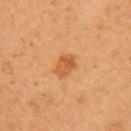Clinical impression:
No biopsy was performed on this lesion — it was imaged during a full skin examination and was not determined to be concerning.
Background:
The patient is a female roughly 35 years of age. A 15 mm crop from a total-body photograph taken for skin-cancer surveillance. On the left upper arm. The lesion-visualizer software estimated an average lesion color of about L≈56 a*≈27 b*≈45 (CIELAB), roughly 10 lightness units darker than nearby skin, and a normalized lesion–skin contrast near 7.5. It also reported a color-variation rating of about 3.5/10 and peripheral color asymmetry of about 1.5. The software also gave a nevus-likeness score of about 85/100.